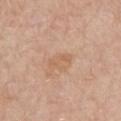notes: no biopsy performed (imaged during a skin exam); patient: male, approximately 75 years of age; acquisition: ~15 mm crop, total-body skin-cancer survey; site: the chest.